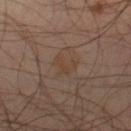biopsy status = total-body-photography surveillance lesion; no biopsy
location = the right thigh
image source = ~15 mm crop, total-body skin-cancer survey
lesion diameter = about 3 mm
subject = male, aged 43 to 47
illumination = cross-polarized
automated lesion analysis = a lesion–skin lightness drop of about 4 and a normalized lesion–skin contrast near 5; border irregularity of about 5.5 on a 0–10 scale, a within-lesion color-variation index near 1/10, and a peripheral color-asymmetry measure near 0.5